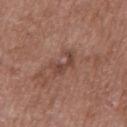Findings:
– notes: imaged on a skin check; not biopsied